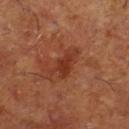The lesion was tiled from a total-body skin photograph and was not biopsied. A male patient, about 65 years old. Captured under cross-polarized illumination. On the right lower leg. The total-body-photography lesion software estimated an area of roughly 7 mm², a shape eccentricity near 0.85, and a symmetry-axis asymmetry near 0.4. It also reported a lesion–skin lightness drop of about 8 and a normalized border contrast of about 7. The lesion's longest dimension is about 4 mm. A 15 mm close-up extracted from a 3D total-body photography capture.